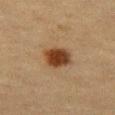Findings:
– biopsy status · catalogued during a skin exam; not biopsied
– subject · female, aged 53–57
– size · ≈3.5 mm
– site · the leg
– tile lighting · cross-polarized illumination
– imaging modality · 15 mm crop, total-body photography